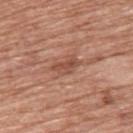Notes:
- anatomic site · the upper back
- illumination · white-light
- patient · female, approximately 60 years of age
- imaging modality · total-body-photography crop, ~15 mm field of view
- lesion diameter · ≈3.5 mm